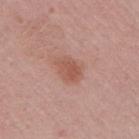Q: Is there a histopathology result?
A: total-body-photography surveillance lesion; no biopsy
Q: Illumination type?
A: white-light
Q: Lesion size?
A: ~3.5 mm (longest diameter)
Q: How was this image acquired?
A: ~15 mm tile from a whole-body skin photo
Q: Who is the patient?
A: female, in their mid- to late 50s
Q: Lesion location?
A: the right upper arm